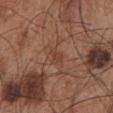The lesion was tiled from a total-body skin photograph and was not biopsied.
The lesion is on the front of the torso.
A male patient aged 53 to 57.
A lesion tile, about 15 mm wide, cut from a 3D total-body photograph.
An algorithmic analysis of the crop reported a lesion color around L≈42 a*≈22 b*≈28 in CIELAB and a lesion–skin lightness drop of about 6. It also reported an automated nevus-likeness rating near 0 out of 100 and lesion-presence confidence of about 95/100.
This is a white-light tile.